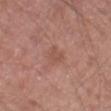This lesion was catalogued during total-body skin photography and was not selected for biopsy. The patient is a male in their mid-50s. A lesion tile, about 15 mm wide, cut from a 3D total-body photograph. From the leg.